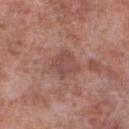follow-up: catalogued during a skin exam; not biopsied | anatomic site: the right lower leg | imaging modality: total-body-photography crop, ~15 mm field of view | patient: male, aged around 55 | illumination: white-light illumination.